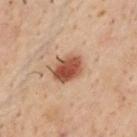notes = catalogued during a skin exam; not biopsied | image source = 15 mm crop, total-body photography | body site = the chest | tile lighting = cross-polarized illumination | subject = male, aged around 50.